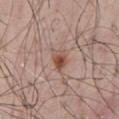notes: catalogued during a skin exam; not biopsied
subject: male, about 60 years old
body site: the chest
size: ≈2.5 mm
imaging modality: total-body-photography crop, ~15 mm field of view